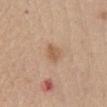follow-up — imaged on a skin check; not biopsied
TBP lesion metrics — a lesion area of about 3.5 mm², a shape eccentricity near 0.75, and a symmetry-axis asymmetry near 0.3
patient — female, aged around 65
illumination — white-light
body site — the abdomen
acquisition — ~15 mm tile from a whole-body skin photo